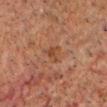Q: Was this lesion biopsied?
A: catalogued during a skin exam; not biopsied
Q: What did automated image analysis measure?
A: an area of roughly 3 mm² and two-axis asymmetry of about 0.4; a mean CIELAB color near L≈34 a*≈18 b*≈26, a lesion–skin lightness drop of about 5, and a normalized border contrast of about 5.5; a border-irregularity rating of about 4/10, a color-variation rating of about 1.5/10, and peripheral color asymmetry of about 0.5; a classifier nevus-likeness of about 0/100 and a detector confidence of about 100 out of 100 that the crop contains a lesion
Q: Lesion location?
A: the head or neck
Q: What lighting was used for the tile?
A: cross-polarized illumination
Q: What are the patient's age and sex?
A: male, aged 58–62
Q: What is the imaging modality?
A: ~15 mm tile from a whole-body skin photo
Q: What is the lesion's diameter?
A: ≈2.5 mm